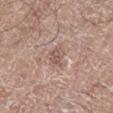workup = no biopsy performed (imaged during a skin exam)
imaging modality = ~15 mm crop, total-body skin-cancer survey
body site = the left lower leg
subject = male, aged 58–62